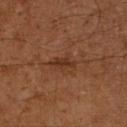Q: Was this lesion biopsied?
A: no biopsy performed (imaged during a skin exam)
Q: What lighting was used for the tile?
A: cross-polarized
Q: What kind of image is this?
A: ~15 mm tile from a whole-body skin photo
Q: Patient demographics?
A: male, about 60 years old
Q: What did automated image analysis measure?
A: a lesion area of about 3.5 mm², a shape eccentricity near 0.9, and a symmetry-axis asymmetry near 0.3; an average lesion color of about L≈30 a*≈20 b*≈28 (CIELAB), about 7 CIELAB-L* units darker than the surrounding skin, and a normalized lesion–skin contrast near 7.5; a border-irregularity index near 4/10 and a within-lesion color-variation index near 1.5/10
Q: Where on the body is the lesion?
A: the leg